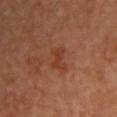Findings:
• follow-up — no biopsy performed (imaged during a skin exam)
• diameter — ~2.5 mm (longest diameter)
• anatomic site — the chest
• subject — male, about 65 years old
• image-analysis metrics — a lesion area of about 4 mm² and an eccentricity of roughly 0.8
• imaging modality — ~15 mm tile from a whole-body skin photo